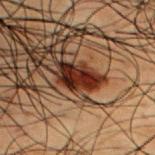{"biopsy_status": "not biopsied; imaged during a skin examination", "patient": {"sex": "male", "age_approx": 50}, "lesion_size": {"long_diameter_mm_approx": 4.5}, "automated_metrics": {"border_irregularity_0_10": 4.5, "color_variation_0_10": 5.0, "peripheral_color_asymmetry": 1.5, "nevus_likeness_0_100": 75, "lesion_detection_confidence_0_100": 100}, "image": {"source": "total-body photography crop", "field_of_view_mm": 15}, "site": "upper back", "lighting": "cross-polarized"}